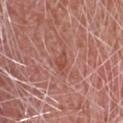{
  "biopsy_status": "not biopsied; imaged during a skin examination",
  "image": {
    "source": "total-body photography crop",
    "field_of_view_mm": 15
  },
  "lighting": "white-light",
  "patient": {
    "sex": "male",
    "age_approx": 65
  },
  "automated_metrics": {
    "cielab_L": 49,
    "cielab_a": 27,
    "cielab_b": 28,
    "vs_skin_darker_L": 7.0,
    "vs_skin_contrast_norm": 6.0,
    "border_irregularity_0_10": 3.5,
    "color_variation_0_10": 0.0,
    "peripheral_color_asymmetry": 0.0
  },
  "site": "head or neck",
  "lesion_size": {
    "long_diameter_mm_approx": 2.5
  }
}